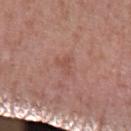The lesion was tiled from a total-body skin photograph and was not biopsied.
The patient is a male aged 73 to 77.
A lesion tile, about 15 mm wide, cut from a 3D total-body photograph.
Located on the leg.